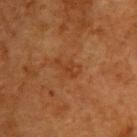biopsy_status: not biopsied; imaged during a skin examination
lesion_size:
  long_diameter_mm_approx: 3.5
lighting: cross-polarized
image:
  source: total-body photography crop
  field_of_view_mm: 15
patient:
  sex: female
  age_approx: 40
site: upper back